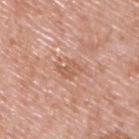biopsy status=imaged on a skin check; not biopsied
size=≈3 mm
subject=male, roughly 50 years of age
image=15 mm crop, total-body photography
lighting=white-light illumination
anatomic site=the upper back
automated lesion analysis=an automated nevus-likeness rating near 0 out of 100 and a detector confidence of about 100 out of 100 that the crop contains a lesion